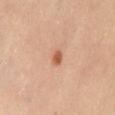No biopsy was performed on this lesion — it was imaged during a full skin examination and was not determined to be concerning. From the abdomen. Imaged with cross-polarized lighting. A 15 mm crop from a total-body photograph taken for skin-cancer surveillance. A female subject, about 35 years old. Approximately 2 mm at its widest.